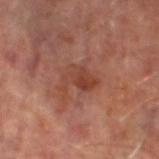No biopsy was performed on this lesion — it was imaged during a full skin examination and was not determined to be concerning. Captured under cross-polarized illumination. The lesion-visualizer software estimated an outline eccentricity of about 0.7 (0 = round, 1 = elongated) and a symmetry-axis asymmetry near 0.5. It also reported border irregularity of about 6 on a 0–10 scale, a within-lesion color-variation index near 4.5/10, and radial color variation of about 1.5. The software also gave a nevus-likeness score of about 0/100 and lesion-presence confidence of about 100/100. A male patient, aged approximately 65. A 15 mm crop from a total-body photograph taken for skin-cancer surveillance. Approximately 4 mm at its widest. Located on the left lower leg.